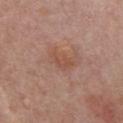Q: Was a biopsy performed?
A: total-body-photography surveillance lesion; no biopsy
Q: What is the imaging modality?
A: ~15 mm tile from a whole-body skin photo
Q: Automated lesion metrics?
A: a lesion area of about 4.5 mm², a shape eccentricity near 0.85, and two-axis asymmetry of about 0.3; an average lesion color of about L≈51 a*≈22 b*≈29 (CIELAB), a lesion–skin lightness drop of about 6, and a lesion-to-skin contrast of about 5.5 (normalized; higher = more distinct); internal color variation of about 3 on a 0–10 scale and peripheral color asymmetry of about 1; an automated nevus-likeness rating near 5 out of 100
Q: Patient demographics?
A: male, approximately 55 years of age
Q: What lighting was used for the tile?
A: white-light
Q: What is the anatomic site?
A: the front of the torso